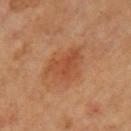{"biopsy_status": "not biopsied; imaged during a skin examination", "patient": {"sex": "female", "age_approx": 65}, "lesion_size": {"long_diameter_mm_approx": 5.0}, "lighting": "cross-polarized", "image": {"source": "total-body photography crop", "field_of_view_mm": 15}, "site": "left upper arm", "automated_metrics": {"area_mm2_approx": 14.0, "eccentricity": 0.65, "shape_asymmetry": 0.3, "cielab_L": 48, "cielab_a": 25, "cielab_b": 35, "vs_skin_darker_L": 7.0}}